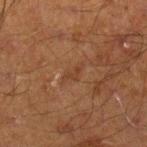{"biopsy_status": "not biopsied; imaged during a skin examination", "site": "right lower leg", "image": {"source": "total-body photography crop", "field_of_view_mm": 15}, "patient": {"sex": "male", "age_approx": 70}, "lighting": "cross-polarized", "lesion_size": {"long_diameter_mm_approx": 2.5}, "automated_metrics": {"cielab_L": 33, "cielab_a": 19, "cielab_b": 27, "vs_skin_contrast_norm": 4.5, "border_irregularity_0_10": 4.5, "nevus_likeness_0_100": 0}}